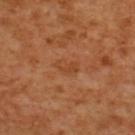{
  "biopsy_status": "not biopsied; imaged during a skin examination",
  "lesion_size": {
    "long_diameter_mm_approx": 3.0
  },
  "site": "upper back",
  "automated_metrics": {
    "area_mm2_approx": 3.0,
    "eccentricity": 0.85,
    "shape_asymmetry": 0.55,
    "border_irregularity_0_10": 5.5,
    "color_variation_0_10": 0.5,
    "peripheral_color_asymmetry": 0.0,
    "nevus_likeness_0_100": 0,
    "lesion_detection_confidence_0_100": 100
  },
  "lighting": "cross-polarized",
  "patient": {
    "sex": "female",
    "age_approx": 55
  },
  "image": {
    "source": "total-body photography crop",
    "field_of_view_mm": 15
  }
}